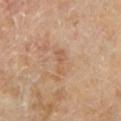TBP lesion metrics = a border-irregularity index near 5.5/10 and a peripheral color-asymmetry measure near 0 | acquisition = 15 mm crop, total-body photography | site = the left lower leg | illumination = cross-polarized | lesion diameter = about 3 mm | subject = male, in their mid-60s.